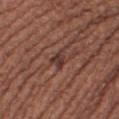The lesion was photographed on a routine skin check and not biopsied; there is no pathology result.
Located on the right thigh.
A 15 mm crop from a total-body photograph taken for skin-cancer surveillance.
The recorded lesion diameter is about 3 mm.
The subject is a female aged around 50.